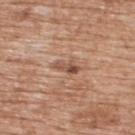Part of a total-body skin-imaging series; this lesion was reviewed on a skin check and was not flagged for biopsy. The subject is a male roughly 60 years of age. This is a white-light tile. The recorded lesion diameter is about 3.5 mm. Automated tile analysis of the lesion measured a lesion color around L≈52 a*≈21 b*≈30 in CIELAB, about 11 CIELAB-L* units darker than the surrounding skin, and a normalized lesion–skin contrast near 7.5. The software also gave an automated nevus-likeness rating near 0 out of 100 and a detector confidence of about 100 out of 100 that the crop contains a lesion. The lesion is on the upper back. A close-up tile cropped from a whole-body skin photograph, about 15 mm across.